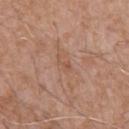lighting=white-light illumination | subject=male, aged 58–62 | imaging modality=15 mm crop, total-body photography | automated lesion analysis=a lesion area of about 2.5 mm², an eccentricity of roughly 0.9, and two-axis asymmetry of about 0.45; an average lesion color of about L≈53 a*≈20 b*≈30 (CIELAB) and roughly 6 lightness units darker than nearby skin; a within-lesion color-variation index near 0/10 and a peripheral color-asymmetry measure near 0 | lesion size=≈2.5 mm | location=the upper back.